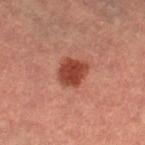notes=catalogued during a skin exam; not biopsied
site=the leg
imaging modality=~15 mm tile from a whole-body skin photo
subject=female, aged 68 to 72